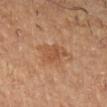Q: Was this lesion biopsied?
A: imaged on a skin check; not biopsied